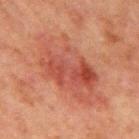Captured during whole-body skin photography for melanoma surveillance; the lesion was not biopsied. A male subject aged around 65. A 15 mm close-up extracted from a 3D total-body photography capture. Located on the chest.